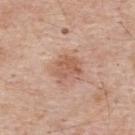biopsy_status: not biopsied; imaged during a skin examination
lesion_size:
  long_diameter_mm_approx: 3.0
image:
  source: total-body photography crop
  field_of_view_mm: 15
lighting: white-light
patient:
  sex: male
  age_approx: 55
site: upper back